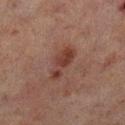The lesion was tiled from a total-body skin photograph and was not biopsied. The recorded lesion diameter is about 4.5 mm. A male subject about 70 years old. Located on the leg. Automated image analysis of the tile measured internal color variation of about 2 on a 0–10 scale and peripheral color asymmetry of about 0.5. The software also gave lesion-presence confidence of about 100/100. A 15 mm close-up extracted from a 3D total-body photography capture. Captured under cross-polarized illumination.